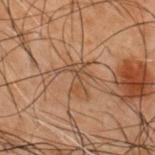Part of a total-body skin-imaging series; this lesion was reviewed on a skin check and was not flagged for biopsy.
The lesion is on the back.
A 15 mm close-up extracted from a 3D total-body photography capture.
A male subject about 50 years old.
Imaged with cross-polarized lighting.